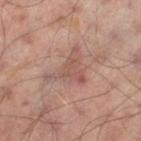Q: Where on the body is the lesion?
A: the left thigh
Q: Patient demographics?
A: male, aged 63–67
Q: What is the imaging modality?
A: ~15 mm tile from a whole-body skin photo